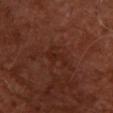Recorded during total-body skin imaging; not selected for excision or biopsy.
The subject is a male aged around 50.
Located on the upper back.
Imaged with cross-polarized lighting.
The lesion-visualizer software estimated a footprint of about 5 mm², an outline eccentricity of about 0.9 (0 = round, 1 = elongated), and two-axis asymmetry of about 0.5. The analysis additionally found roughly 5 lightness units darker than nearby skin and a normalized lesion–skin contrast near 5.5. It also reported a border-irregularity index near 6.5/10, internal color variation of about 3 on a 0–10 scale, and peripheral color asymmetry of about 1. It also reported a classifier nevus-likeness of about 0/100.
A 15 mm crop from a total-body photograph taken for skin-cancer surveillance.
The lesion's longest dimension is about 4 mm.